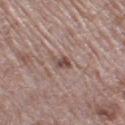  biopsy_status: not biopsied; imaged during a skin examination
  lighting: white-light
  site: left thigh
  image:
    source: total-body photography crop
    field_of_view_mm: 15
  lesion_size:
    long_diameter_mm_approx: 2.5
  patient:
    sex: male
    age_approx: 70